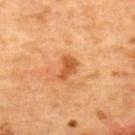This lesion was catalogued during total-body skin photography and was not selected for biopsy. Approximately 3 mm at its widest. The lesion is on the back. A female subject about 70 years old. A 15 mm close-up tile from a total-body photography series done for melanoma screening. Automated image analysis of the tile measured a classifier nevus-likeness of about 55/100 and a lesion-detection confidence of about 100/100. Imaged with cross-polarized lighting.